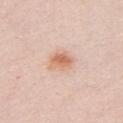Impression: This lesion was catalogued during total-body skin photography and was not selected for biopsy. Background: A female patient aged approximately 40. On the chest. A 15 mm crop from a total-body photograph taken for skin-cancer surveillance. This is a white-light tile.